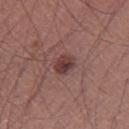Q: Was a biopsy performed?
A: total-body-photography surveillance lesion; no biopsy
Q: How large is the lesion?
A: ≈2.5 mm
Q: Patient demographics?
A: male, roughly 45 years of age
Q: What is the imaging modality?
A: ~15 mm tile from a whole-body skin photo
Q: Lesion location?
A: the left thigh
Q: How was the tile lit?
A: white-light illumination
Q: Automated lesion metrics?
A: a border-irregularity rating of about 1.5/10 and a color-variation rating of about 4/10; a nevus-likeness score of about 80/100